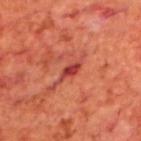Assessment: This lesion was catalogued during total-body skin photography and was not selected for biopsy. Background: A male patient aged 68–72. A 15 mm close-up tile from a total-body photography series done for melanoma screening. This is a cross-polarized tile. The lesion is located on the upper back. Automated tile analysis of the lesion measured an eccentricity of roughly 0.9 and a symmetry-axis asymmetry near 0.3. The analysis additionally found a border-irregularity rating of about 3/10, a color-variation rating of about 1/10, and a peripheral color-asymmetry measure near 0.5. About 3 mm across.